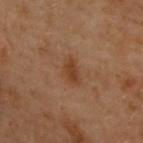Q: Was a biopsy performed?
A: catalogued during a skin exam; not biopsied
Q: What is the imaging modality?
A: 15 mm crop, total-body photography
Q: How was the tile lit?
A: cross-polarized
Q: How large is the lesion?
A: about 3.5 mm
Q: What are the patient's age and sex?
A: female, approximately 60 years of age
Q: Lesion location?
A: the upper back
Q: What did automated image analysis measure?
A: a lesion area of about 5.5 mm², an eccentricity of roughly 0.75, and a shape-asymmetry score of about 0.3 (0 = symmetric); border irregularity of about 2.5 on a 0–10 scale, a color-variation rating of about 2/10, and a peripheral color-asymmetry measure near 0.5; a nevus-likeness score of about 60/100